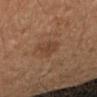No biopsy was performed on this lesion — it was imaged during a full skin examination and was not determined to be concerning. Automated image analysis of the tile measured an outline eccentricity of about 0.55 (0 = round, 1 = elongated) and a shape-asymmetry score of about 0.3 (0 = symmetric). The analysis additionally found an average lesion color of about L≈43 a*≈20 b*≈31 (CIELAB), a lesion–skin lightness drop of about 7, and a normalized border contrast of about 5.5. And it measured a color-variation rating of about 2/10 and peripheral color asymmetry of about 0.5. The software also gave a classifier nevus-likeness of about 15/100 and a detector confidence of about 100 out of 100 that the crop contains a lesion. This is a cross-polarized tile. A female patient aged around 40. About 3 mm across. The lesion is on the left arm. A lesion tile, about 15 mm wide, cut from a 3D total-body photograph.